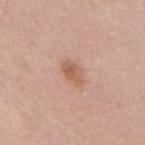<record>
  <lesion_size>
    <long_diameter_mm_approx>3.0</long_diameter_mm_approx>
  </lesion_size>
  <site>upper back</site>
  <lighting>white-light</lighting>
  <image>
    <source>total-body photography crop</source>
    <field_of_view_mm>15</field_of_view_mm>
  </image>
  <automated_metrics>
    <area_mm2_approx>4.5</area_mm2_approx>
    <shape_asymmetry>0.15</shape_asymmetry>
    <cielab_L>59</cielab_L>
    <cielab_a>22</cielab_a>
    <cielab_b>30</cielab_b>
    <vs_skin_contrast_norm>6.5</vs_skin_contrast_norm>
    <nevus_likeness_0_100>65</nevus_likeness_0_100>
    <lesion_detection_confidence_0_100>100</lesion_detection_confidence_0_100>
  </automated_metrics>
  <patient>
    <sex>female</sex>
    <age_approx>30</age_approx>
  </patient>
</record>